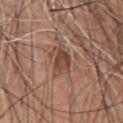Q: Is there a histopathology result?
A: total-body-photography surveillance lesion; no biopsy
Q: What is the imaging modality?
A: 15 mm crop, total-body photography
Q: Patient demographics?
A: male, approximately 60 years of age
Q: Automated lesion metrics?
A: a footprint of about 5.5 mm², an outline eccentricity of about 0.85 (0 = round, 1 = elongated), and a symmetry-axis asymmetry near 0.45; an average lesion color of about L≈45 a*≈21 b*≈27 (CIELAB) and about 10 CIELAB-L* units darker than the surrounding skin; a border-irregularity rating of about 5/10, internal color variation of about 4 on a 0–10 scale, and radial color variation of about 1.5; an automated nevus-likeness rating near 5 out of 100
Q: What lighting was used for the tile?
A: white-light
Q: Where on the body is the lesion?
A: the chest